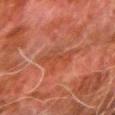follow-up: catalogued during a skin exam; not biopsied
imaging modality: 15 mm crop, total-body photography
site: the right forearm
subject: male, aged approximately 80